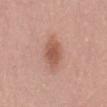About 4 mm across.
Imaged with white-light lighting.
A 15 mm crop from a total-body photograph taken for skin-cancer surveillance.
The patient is a female aged approximately 65.
The lesion is located on the back.
The total-body-photography lesion software estimated a border-irregularity index near 1.5/10, internal color variation of about 2.5 on a 0–10 scale, and radial color variation of about 0.5. The software also gave a nevus-likeness score of about 95/100.